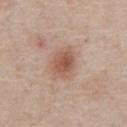Clinical impression:
No biopsy was performed on this lesion — it was imaged during a full skin examination and was not determined to be concerning.
Acquisition and patient details:
Automated tile analysis of the lesion measured a shape eccentricity near 0.65. And it measured a border-irregularity rating of about 2/10, a within-lesion color-variation index near 5/10, and a peripheral color-asymmetry measure near 1.5. The tile uses white-light illumination. On the chest. A male subject roughly 60 years of age. A roughly 15 mm field-of-view crop from a total-body skin photograph.